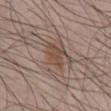• workup: total-body-photography surveillance lesion; no biopsy
• subject: male, aged approximately 50
• lesion size: about 3.5 mm
• image source: total-body-photography crop, ~15 mm field of view
• location: the chest
• lighting: white-light illumination
• automated lesion analysis: a footprint of about 6.5 mm²; a nevus-likeness score of about 45/100 and lesion-presence confidence of about 100/100